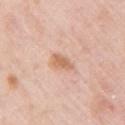Assessment: No biopsy was performed on this lesion — it was imaged during a full skin examination and was not determined to be concerning. Image and clinical context: A female patient, about 65 years old. Captured under white-light illumination. From the right upper arm. Approximately 3 mm at its widest. Cropped from a total-body skin-imaging series; the visible field is about 15 mm.